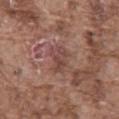| feature | finding |
|---|---|
| workup | no biopsy performed (imaged during a skin exam) |
| patient | male, aged approximately 75 |
| anatomic site | the abdomen |
| image source | ~15 mm tile from a whole-body skin photo |